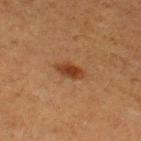notes: no biopsy performed (imaged during a skin exam)
site: the right thigh
subject: female, aged approximately 50
lesion diameter: about 3 mm
illumination: cross-polarized illumination
acquisition: 15 mm crop, total-body photography